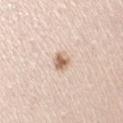{"biopsy_status": "not biopsied; imaged during a skin examination", "site": "right upper arm", "lesion_size": {"long_diameter_mm_approx": 2.5}, "image": {"source": "total-body photography crop", "field_of_view_mm": 15}, "automated_metrics": {"eccentricity": 0.7, "shape_asymmetry": 0.15, "border_irregularity_0_10": 1.5, "color_variation_0_10": 3.0, "peripheral_color_asymmetry": 1.0, "nevus_likeness_0_100": 95}, "patient": {"sex": "female", "age_approx": 40}, "lighting": "white-light"}